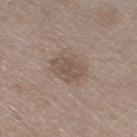<lesion>
<biopsy_status>not biopsied; imaged during a skin examination</biopsy_status>
<image>
  <source>total-body photography crop</source>
  <field_of_view_mm>15</field_of_view_mm>
</image>
<patient>
  <sex>male</sex>
  <age_approx>50</age_approx>
</patient>
<site>leg</site>
</lesion>